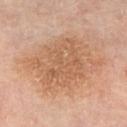Recorded during total-body skin imaging; not selected for excision or biopsy.